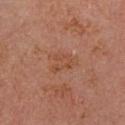  biopsy_status: not biopsied; imaged during a skin examination
  image:
    source: total-body photography crop
    field_of_view_mm: 15
  patient:
    sex: female
    age_approx: 60
  lighting: cross-polarized
  automated_metrics:
    border_irregularity_0_10: 5.0
    color_variation_0_10: 2.0
    peripheral_color_asymmetry: 0.5
    lesion_detection_confidence_0_100: 100
  site: head or neck
  lesion_size:
    long_diameter_mm_approx: 3.5